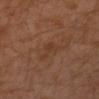workup=catalogued during a skin exam; not biopsied
tile lighting=cross-polarized
subject=male, in their 30s
site=the left forearm
lesion diameter=≈3 mm
image-analysis metrics=a lesion color around L≈36 a*≈19 b*≈30 in CIELAB, roughly 4 lightness units darker than nearby skin, and a normalized lesion–skin contrast near 5; border irregularity of about 5.5 on a 0–10 scale, a within-lesion color-variation index near 1.5/10, and a peripheral color-asymmetry measure near 0.5; an automated nevus-likeness rating near 0 out of 100 and a detector confidence of about 100 out of 100 that the crop contains a lesion
image source=total-body-photography crop, ~15 mm field of view